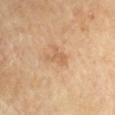Imaged during a routine full-body skin examination; the lesion was not biopsied and no histopathology is available. Longest diameter approximately 3 mm. Automated tile analysis of the lesion measured a lesion area of about 4 mm². The analysis additionally found a border-irregularity index near 6/10, a within-lesion color-variation index near 1.5/10, and peripheral color asymmetry of about 0.5. And it measured a classifier nevus-likeness of about 10/100. The lesion is on the arm. Cropped from a total-body skin-imaging series; the visible field is about 15 mm. Imaged with cross-polarized lighting. A male patient, aged 68 to 72.